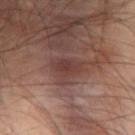Clinical summary:
On the abdomen. The tile uses cross-polarized illumination. About 3 mm across. The total-body-photography lesion software estimated an outline eccentricity of about 0.8 (0 = round, 1 = elongated) and a shape-asymmetry score of about 0.2 (0 = symmetric). It also reported an automated nevus-likeness rating near 0 out of 100 and lesion-presence confidence of about 95/100. A male subject in their 40s. A close-up tile cropped from a whole-body skin photograph, about 15 mm across.